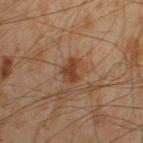Captured under cross-polarized illumination.
Longest diameter approximately 3 mm.
Automated tile analysis of the lesion measured an area of roughly 4 mm² and a shape-asymmetry score of about 0.25 (0 = symmetric). The software also gave a border-irregularity rating of about 2.5/10. It also reported a lesion-detection confidence of about 100/100.
A male patient, in their mid- to late 40s.
A close-up tile cropped from a whole-body skin photograph, about 15 mm across.
The lesion is located on the left lower leg.